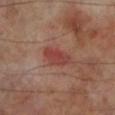| field | value |
|---|---|
| illumination | cross-polarized |
| anatomic site | the left lower leg |
| size | ~4 mm (longest diameter) |
| subject | male, roughly 70 years of age |
| image source | ~15 mm tile from a whole-body skin photo |